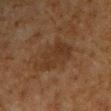<lesion>
  <biopsy_status>not biopsied; imaged during a skin examination</biopsy_status>
  <image>
    <source>total-body photography crop</source>
    <field_of_view_mm>15</field_of_view_mm>
  </image>
  <patient>
    <sex>male</sex>
    <age_approx>60</age_approx>
  </patient>
  <site>chest</site>
</lesion>